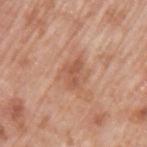| feature | finding |
|---|---|
| follow-up | total-body-photography surveillance lesion; no biopsy |
| diameter | about 3.5 mm |
| patient | male, aged around 70 |
| site | the left upper arm |
| TBP lesion metrics | a footprint of about 4.5 mm², a shape eccentricity near 0.8, and two-axis asymmetry of about 0.45; an average lesion color of about L≈55 a*≈24 b*≈32 (CIELAB) and a normalized lesion–skin contrast near 6; border irregularity of about 5 on a 0–10 scale and a peripheral color-asymmetry measure near 0.5; an automated nevus-likeness rating near 0 out of 100 and a lesion-detection confidence of about 100/100 |
| image | total-body-photography crop, ~15 mm field of view |
| lighting | white-light |